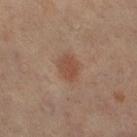This lesion was catalogued during total-body skin photography and was not selected for biopsy.
The lesion is located on the left leg.
A female subject, aged 63 to 67.
The recorded lesion diameter is about 3 mm.
This image is a 15 mm lesion crop taken from a total-body photograph.
Imaged with cross-polarized lighting.